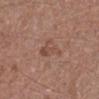About 4 mm across.
A male subject, aged around 60.
The lesion is on the left lower leg.
This is a white-light tile.
A close-up tile cropped from a whole-body skin photograph, about 15 mm across.